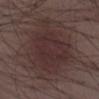Q: Is there a histopathology result?
A: no biopsy performed (imaged during a skin exam)
Q: What is the imaging modality?
A: 15 mm crop, total-body photography
Q: What is the anatomic site?
A: the right lower leg
Q: What are the patient's age and sex?
A: male, about 40 years old
Q: Lesion size?
A: ≈8 mm
Q: Illumination type?
A: white-light